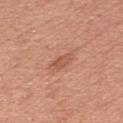The lesion was tiled from a total-body skin photograph and was not biopsied. A female subject in their 50s. A region of skin cropped from a whole-body photographic capture, roughly 15 mm wide. Approximately 2.5 mm at its widest. This is a white-light tile. On the arm.